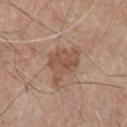| key | value |
|---|---|
| body site | the chest |
| size | about 5 mm |
| subject | male, roughly 80 years of age |
| image source | total-body-photography crop, ~15 mm field of view |
| tile lighting | white-light illumination |